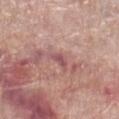Q: Was this lesion biopsied?
A: catalogued during a skin exam; not biopsied
Q: Who is the patient?
A: female, in their 60s
Q: What is the anatomic site?
A: the left lower leg
Q: How large is the lesion?
A: about 2.5 mm
Q: How was this image acquired?
A: ~15 mm tile from a whole-body skin photo
Q: Illumination type?
A: white-light illumination